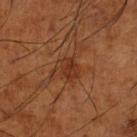follow-up=imaged on a skin check; not biopsied | tile lighting=cross-polarized | size=~3 mm (longest diameter) | imaging modality=~15 mm crop, total-body skin-cancer survey | subject=male, in their mid- to late 60s | site=the left lower leg | TBP lesion metrics=a lesion color around L≈35 a*≈25 b*≈33 in CIELAB, about 7 CIELAB-L* units darker than the surrounding skin, and a normalized border contrast of about 6.5.